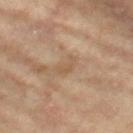notes=total-body-photography surveillance lesion; no biopsy
lesion size=about 2.5 mm
illumination=cross-polarized illumination
imaging modality=15 mm crop, total-body photography
site=the left thigh
patient=female, about 75 years old